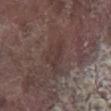Q: Was a biopsy performed?
A: no biopsy performed (imaged during a skin exam)
Q: Where on the body is the lesion?
A: the right lower leg
Q: Lesion size?
A: ~4 mm (longest diameter)
Q: Patient demographics?
A: male, in their mid- to late 70s
Q: What lighting was used for the tile?
A: white-light illumination
Q: How was this image acquired?
A: total-body-photography crop, ~15 mm field of view